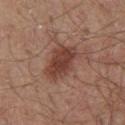Q: Was this lesion biopsied?
A: no biopsy performed (imaged during a skin exam)
Q: What did automated image analysis measure?
A: lesion-presence confidence of about 100/100
Q: Patient demographics?
A: male, roughly 55 years of age
Q: How was the tile lit?
A: white-light
Q: Lesion location?
A: the chest
Q: How large is the lesion?
A: ~5.5 mm (longest diameter)
Q: What is the imaging modality?
A: total-body-photography crop, ~15 mm field of view